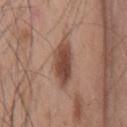site = the chest | tile lighting = white-light | image-analysis metrics = a border-irregularity rating of about 2/10, a within-lesion color-variation index near 4.5/10, and peripheral color asymmetry of about 1.5; a classifier nevus-likeness of about 95/100 and a detector confidence of about 100 out of 100 that the crop contains a lesion | patient = male, aged 53–57 | image source = total-body-photography crop, ~15 mm field of view | lesion diameter = about 5.5 mm.